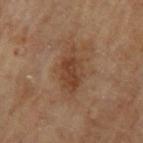Cropped from a total-body skin-imaging series; the visible field is about 15 mm. From the left upper arm. A male patient, aged approximately 70.